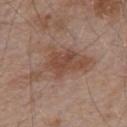Part of a total-body skin-imaging series; this lesion was reviewed on a skin check and was not flagged for biopsy. A male subject, about 55 years old. A roughly 15 mm field-of-view crop from a total-body skin photograph. Located on the upper back. Longest diameter approximately 7.5 mm.